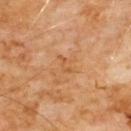biopsy status — imaged on a skin check; not biopsied | site — the chest | subject — male, about 60 years old | acquisition — ~15 mm tile from a whole-body skin photo | diameter — ≈2.5 mm | automated metrics — a shape eccentricity near 0.9 and a symmetry-axis asymmetry near 0.5; an automated nevus-likeness rating near 0 out of 100 and lesion-presence confidence of about 100/100.